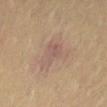Notes:
- workup · no biopsy performed (imaged during a skin exam)
- tile lighting · cross-polarized
- body site · the abdomen
- patient · female, aged around 80
- image source · total-body-photography crop, ~15 mm field of view